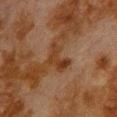Assessment:
Part of a total-body skin-imaging series; this lesion was reviewed on a skin check and was not flagged for biopsy.
Acquisition and patient details:
Cropped from a total-body skin-imaging series; the visible field is about 15 mm. This is a cross-polarized tile. The lesion is on the back. The total-body-photography lesion software estimated a border-irregularity index near 6/10 and a within-lesion color-variation index near 3.5/10. And it measured an automated nevus-likeness rating near 0 out of 100 and a detector confidence of about 100 out of 100 that the crop contains a lesion. A male patient aged around 80.